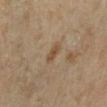site: the left lower leg
tile lighting: cross-polarized illumination
subject: male, in their mid- to late 60s
acquisition: total-body-photography crop, ~15 mm field of view
diameter: ~3 mm (longest diameter)
automated metrics: an eccentricity of roughly 0.9 and a shape-asymmetry score of about 0.25 (0 = symmetric); border irregularity of about 3 on a 0–10 scale, a color-variation rating of about 1/10, and peripheral color asymmetry of about 0; a classifier nevus-likeness of about 0/100 and a detector confidence of about 100 out of 100 that the crop contains a lesion